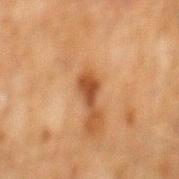Part of a total-body skin-imaging series; this lesion was reviewed on a skin check and was not flagged for biopsy. The subject is a male approximately 60 years of age. Located on the back. A region of skin cropped from a whole-body photographic capture, roughly 15 mm wide. Imaged with cross-polarized lighting. Automated image analysis of the tile measured a footprint of about 3 mm², an outline eccentricity of about 0.85 (0 = round, 1 = elongated), and two-axis asymmetry of about 0.25. And it measured a border-irregularity index near 3/10.